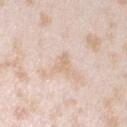{"biopsy_status": "not biopsied; imaged during a skin examination", "image": {"source": "total-body photography crop", "field_of_view_mm": 15}, "site": "left upper arm", "patient": {"sex": "female", "age_approx": 25}, "automated_metrics": {"area_mm2_approx": 3.0, "shape_asymmetry": 0.45, "cielab_L": 72, "cielab_a": 15, "cielab_b": 30, "vs_skin_contrast_norm": 5.0}, "lesion_size": {"long_diameter_mm_approx": 2.5}}